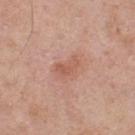Q: Was this lesion biopsied?
A: no biopsy performed (imaged during a skin exam)
Q: What are the patient's age and sex?
A: male, approximately 55 years of age
Q: How large is the lesion?
A: ~3 mm (longest diameter)
Q: What is the imaging modality?
A: 15 mm crop, total-body photography
Q: What is the anatomic site?
A: the upper back